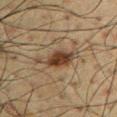Assessment: Captured during whole-body skin photography for melanoma surveillance; the lesion was not biopsied. Image and clinical context: A lesion tile, about 15 mm wide, cut from a 3D total-body photograph. The patient is a male roughly 60 years of age. Located on the front of the torso.